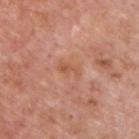{
  "biopsy_status": "not biopsied; imaged during a skin examination",
  "patient": {
    "sex": "male",
    "age_approx": 75
  },
  "image": {
    "source": "total-body photography crop",
    "field_of_view_mm": 15
  },
  "site": "upper back",
  "lighting": "white-light",
  "lesion_size": {
    "long_diameter_mm_approx": 3.0
  }
}